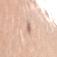Part of a total-body skin-imaging series; this lesion was reviewed on a skin check and was not flagged for biopsy. A region of skin cropped from a whole-body photographic capture, roughly 15 mm wide. The lesion-visualizer software estimated a lesion area of about 3 mm², a shape eccentricity near 0.9, and two-axis asymmetry of about 0.3. The analysis additionally found a mean CIELAB color near L≈69 a*≈18 b*≈28 and a lesion–skin lightness drop of about 9. On the head or neck. The subject is a female aged approximately 30. The tile uses white-light illumination. Longest diameter approximately 2.5 mm.